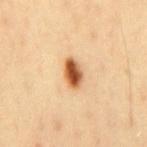Clinical impression:
Recorded during total-body skin imaging; not selected for excision or biopsy.
Image and clinical context:
Measured at roughly 3.5 mm in maximum diameter. The lesion is on the back. The tile uses cross-polarized illumination. The total-body-photography lesion software estimated a footprint of about 6 mm², an outline eccentricity of about 0.8 (0 = round, 1 = elongated), and a shape-asymmetry score of about 0.15 (0 = symmetric). It also reported a normalized lesion–skin contrast near 12.5. A close-up tile cropped from a whole-body skin photograph, about 15 mm across. A male patient about 40 years old.